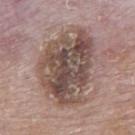Q: Is there a histopathology result?
A: total-body-photography surveillance lesion; no biopsy
Q: Where on the body is the lesion?
A: the upper back
Q: Lesion size?
A: ~9 mm (longest diameter)
Q: How was the tile lit?
A: white-light illumination
Q: What kind of image is this?
A: 15 mm crop, total-body photography
Q: Who is the patient?
A: male, aged approximately 85 or older